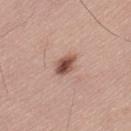Acquisition and patient details: The tile uses white-light illumination. A male patient, aged 63 to 67. The total-body-photography lesion software estimated an eccentricity of roughly 0.7 and two-axis asymmetry of about 0.2. A lesion tile, about 15 mm wide, cut from a 3D total-body photograph. The lesion's longest dimension is about 3 mm. On the lower back.